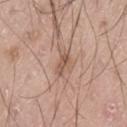Recorded during total-body skin imaging; not selected for excision or biopsy. A 15 mm close-up extracted from a 3D total-body photography capture. A male subject, about 20 years old. This is a white-light tile. The lesion is on the left thigh.